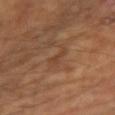Recorded during total-body skin imaging; not selected for excision or biopsy. A 15 mm close-up tile from a total-body photography series done for melanoma screening. The lesion is located on the arm. A male subject, aged 63 to 67.